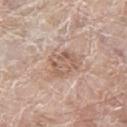Context:
The tile uses white-light illumination. Longest diameter approximately 3.5 mm. A male patient in their 80s. A close-up tile cropped from a whole-body skin photograph, about 15 mm across. On the left thigh.